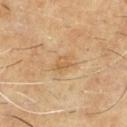notes — catalogued during a skin exam; not biopsied
site — the chest
lighting — cross-polarized illumination
automated lesion analysis — a footprint of about 2.5 mm², an outline eccentricity of about 0.55 (0 = round, 1 = elongated), and two-axis asymmetry of about 0.3; a lesion-detection confidence of about 100/100
image source — 15 mm crop, total-body photography
size — about 2 mm
subject — male, aged around 60